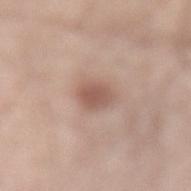Part of a total-body skin-imaging series; this lesion was reviewed on a skin check and was not flagged for biopsy.
A 15 mm crop from a total-body photograph taken for skin-cancer surveillance.
A male subject, aged 58–62.
Automated tile analysis of the lesion measured a lesion–skin lightness drop of about 10. The software also gave a border-irregularity rating of about 2/10. And it measured lesion-presence confidence of about 100/100.
The lesion is on the lower back.